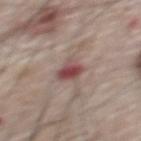Clinical impression:
Imaged during a routine full-body skin examination; the lesion was not biopsied and no histopathology is available.
Context:
A close-up tile cropped from a whole-body skin photograph, about 15 mm across. The patient is a male aged 63 to 67. The lesion is on the upper back. Longest diameter approximately 3 mm.